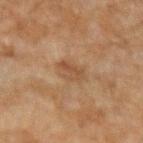size: ~3.5 mm (longest diameter); body site: the left forearm; imaging modality: total-body-photography crop, ~15 mm field of view; lighting: cross-polarized illumination; patient: male, aged around 45.